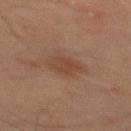No biopsy was performed on this lesion — it was imaged during a full skin examination and was not determined to be concerning. About 3.5 mm across. A male subject aged 43–47. Cropped from a total-body skin-imaging series; the visible field is about 15 mm. The tile uses cross-polarized illumination. From the mid back.